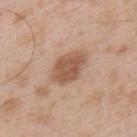biopsy_status: not biopsied; imaged during a skin examination
site: upper back
lesion_size:
  long_diameter_mm_approx: 5.0
patient:
  sex: male
  age_approx: 50
image:
  source: total-body photography crop
  field_of_view_mm: 15